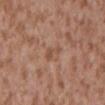Q: Was this lesion biopsied?
A: total-body-photography surveillance lesion; no biopsy
Q: How was the tile lit?
A: white-light
Q: What is the imaging modality?
A: 15 mm crop, total-body photography
Q: Patient demographics?
A: male, about 45 years old
Q: How large is the lesion?
A: about 3 mm
Q: What is the anatomic site?
A: the mid back